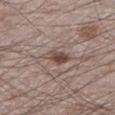Impression: No biopsy was performed on this lesion — it was imaged during a full skin examination and was not determined to be concerning. Clinical summary: Automated tile analysis of the lesion measured a border-irregularity index near 2/10, internal color variation of about 5.5 on a 0–10 scale, and radial color variation of about 2. The analysis additionally found an automated nevus-likeness rating near 90 out of 100. The lesion is on the left lower leg. The subject is a male aged around 60. The tile uses white-light illumination. A 15 mm close-up extracted from a 3D total-body photography capture. Measured at roughly 3.5 mm in maximum diameter.